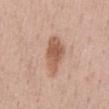{"biopsy_status": "not biopsied; imaged during a skin examination", "patient": {"sex": "female", "age_approx": 45}, "site": "front of the torso", "image": {"source": "total-body photography crop", "field_of_view_mm": 15}, "automated_metrics": {"eccentricity": 0.9, "shape_asymmetry": 0.2, "cielab_L": 58, "cielab_a": 21, "cielab_b": 30, "vs_skin_darker_L": 12.0, "border_irregularity_0_10": 3.0}, "lighting": "white-light"}